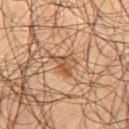<tbp_lesion>
<biopsy_status>not biopsied; imaged during a skin examination</biopsy_status>
<image>
  <source>total-body photography crop</source>
  <field_of_view_mm>15</field_of_view_mm>
</image>
<patient>
  <sex>male</sex>
  <age_approx>55</age_approx>
</patient>
<site>right thigh</site>
</tbp_lesion>